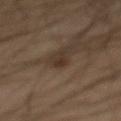biopsy status = imaged on a skin check; not biopsied
acquisition = ~15 mm tile from a whole-body skin photo
subject = male, approximately 65 years of age
location = the abdomen